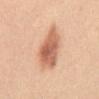{"biopsy_status": "not biopsied; imaged during a skin examination", "lesion_size": {"long_diameter_mm_approx": 6.0}, "automated_metrics": {"border_irregularity_0_10": 2.5, "peripheral_color_asymmetry": 1.5}, "image": {"source": "total-body photography crop", "field_of_view_mm": 15}, "patient": {"sex": "female", "age_approx": 30}, "site": "abdomen"}